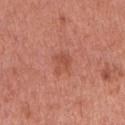notes=imaged on a skin check; not biopsied | anatomic site=the arm | automated metrics=a lesion color around L≈52 a*≈29 b*≈32 in CIELAB, roughly 7 lightness units darker than nearby skin, and a normalized lesion–skin contrast near 5.5; an automated nevus-likeness rating near 15 out of 100 and a detector confidence of about 100 out of 100 that the crop contains a lesion | image source=15 mm crop, total-body photography | lesion size=~2.5 mm (longest diameter) | illumination=white-light | patient=female, in their 50s.